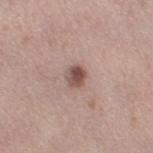biopsy status: catalogued during a skin exam; not biopsied
lesion size: about 2.5 mm
illumination: white-light
imaging modality: ~15 mm tile from a whole-body skin photo
patient: female, approximately 40 years of age
site: the right thigh